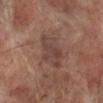Captured during whole-body skin photography for melanoma surveillance; the lesion was not biopsied. The lesion is located on the left lower leg. A male subject aged 68–72. The lesion-visualizer software estimated a lesion area of about 11 mm² and a symmetry-axis asymmetry near 0.3. The software also gave roughly 7 lightness units darker than nearby skin and a normalized lesion–skin contrast near 6. And it measured a border-irregularity index near 3/10 and internal color variation of about 3.5 on a 0–10 scale. The lesion's longest dimension is about 4.5 mm. A 15 mm crop from a total-body photograph taken for skin-cancer surveillance. Imaged with white-light lighting.